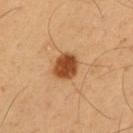The lesion was tiled from a total-body skin photograph and was not biopsied.
On the arm.
A male subject, approximately 55 years of age.
This image is a 15 mm lesion crop taken from a total-body photograph.
About 3 mm across.
The tile uses cross-polarized illumination.